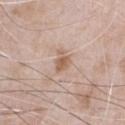Case summary:
• biopsy status — no biopsy performed (imaged during a skin exam)
• imaging modality — total-body-photography crop, ~15 mm field of view
• subject — male, in their 50s
• anatomic site — the chest